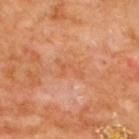follow-up — catalogued during a skin exam; not biopsied
image — ~15 mm crop, total-body skin-cancer survey
subject — male, aged approximately 70
anatomic site — the upper back
TBP lesion metrics — an average lesion color of about L≈57 a*≈27 b*≈38 (CIELAB)
diameter — ≈3.5 mm
lighting — cross-polarized